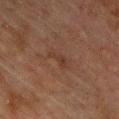biopsy_status: not biopsied; imaged during a skin examination
lesion_size:
  long_diameter_mm_approx: 2.5
automated_metrics:
  lesion_detection_confidence_0_100: 100
site: chest
patient:
  sex: male
  age_approx: 75
lighting: cross-polarized
image:
  source: total-body photography crop
  field_of_view_mm: 15